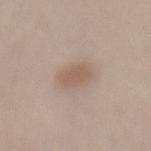notes — catalogued during a skin exam; not biopsied
acquisition — ~15 mm tile from a whole-body skin photo
body site — the lower back
patient — male, aged approximately 55